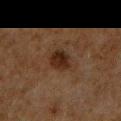follow-up = total-body-photography surveillance lesion; no biopsy.